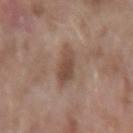follow-up = total-body-photography surveillance lesion; no biopsy
image-analysis metrics = a lesion area of about 8.5 mm², a shape eccentricity near 0.75, and a symmetry-axis asymmetry near 0.2; an automated nevus-likeness rating near 15 out of 100
acquisition = 15 mm crop, total-body photography
subject = female, aged approximately 60
illumination = white-light illumination
lesion size = about 4 mm
anatomic site = the left forearm